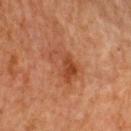Clinical impression: No biopsy was performed on this lesion — it was imaged during a full skin examination and was not determined to be concerning. Context: On the upper back. A female subject, aged around 70. About 4.5 mm across. A region of skin cropped from a whole-body photographic capture, roughly 15 mm wide.